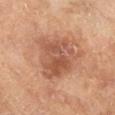{
  "biopsy_status": "not biopsied; imaged during a skin examination",
  "automated_metrics": {
    "cielab_L": 52,
    "cielab_a": 23,
    "cielab_b": 31,
    "vs_skin_contrast_norm": 7.0,
    "nevus_likeness_0_100": 15,
    "lesion_detection_confidence_0_100": 100
  },
  "patient": {
    "sex": "male",
    "age_approx": 65
  },
  "site": "right lower leg",
  "image": {
    "source": "total-body photography crop",
    "field_of_view_mm": 15
  },
  "lesion_size": {
    "long_diameter_mm_approx": 6.0
  },
  "lighting": "cross-polarized"
}